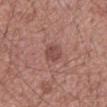notes: no biopsy performed (imaged during a skin exam); location: the mid back; image: total-body-photography crop, ~15 mm field of view; tile lighting: white-light; subject: male, aged 53–57.